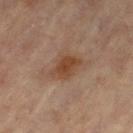notes: imaged on a skin check; not biopsied
tile lighting: cross-polarized illumination
automated metrics: an area of roughly 11 mm², a shape eccentricity near 0.75, and a shape-asymmetry score of about 0.35 (0 = symmetric); a border-irregularity index near 4.5/10, internal color variation of about 3.5 on a 0–10 scale, and a peripheral color-asymmetry measure near 1; a detector confidence of about 100 out of 100 that the crop contains a lesion
size: ≈4.5 mm
patient: female, about 65 years old
image source: 15 mm crop, total-body photography
location: the right leg